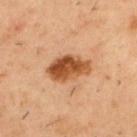Assessment: Captured during whole-body skin photography for melanoma surveillance; the lesion was not biopsied. Acquisition and patient details: From the upper back. The recorded lesion diameter is about 5 mm. The patient is a male aged around 55. The tile uses cross-polarized illumination. This image is a 15 mm lesion crop taken from a total-body photograph.